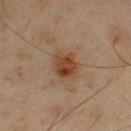  biopsy_status: not biopsied; imaged during a skin examination
  patient:
    sex: male
    age_approx: 45
  automated_metrics:
    area_mm2_approx: 7.5
    eccentricity: 0.65
    shape_asymmetry: 0.2
    cielab_L: 33
    cielab_a: 17
    cielab_b: 27
    vs_skin_darker_L: 9.0
    vs_skin_contrast_norm: 9.0
    border_irregularity_0_10: 2.5
    peripheral_color_asymmetry: 2.0
  lesion_size:
    long_diameter_mm_approx: 3.5
  site: left thigh
  image:
    source: total-body photography crop
    field_of_view_mm: 15